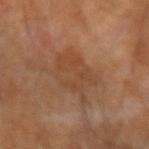{"biopsy_status": "not biopsied; imaged during a skin examination", "lesion_size": {"long_diameter_mm_approx": 5.0}, "image": {"source": "total-body photography crop", "field_of_view_mm": 15}, "site": "right forearm", "automated_metrics": {"area_mm2_approx": 13.0, "shape_asymmetry": 0.35, "color_variation_0_10": 3.0, "peripheral_color_asymmetry": 1.0, "lesion_detection_confidence_0_100": 100}, "patient": {"sex": "male", "age_approx": 65}, "lighting": "cross-polarized"}